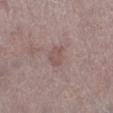No biopsy was performed on this lesion — it was imaged during a full skin examination and was not determined to be concerning.
The subject is a male aged 78 to 82.
Approximately 3 mm at its widest.
A close-up tile cropped from a whole-body skin photograph, about 15 mm across.
This is a white-light tile.
The lesion is on the right lower leg.